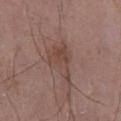No biopsy was performed on this lesion — it was imaged during a full skin examination and was not determined to be concerning.